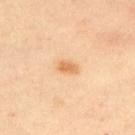biopsy status: imaged on a skin check; not biopsied | subject: male, aged around 45 | tile lighting: cross-polarized | location: the upper back | acquisition: 15 mm crop, total-body photography | TBP lesion metrics: a footprint of about 3 mm², a shape eccentricity near 0.85, and a symmetry-axis asymmetry near 0.3; a border-irregularity index near 2.5/10, a color-variation rating of about 1.5/10, and radial color variation of about 0.5; an automated nevus-likeness rating near 90 out of 100 and a detector confidence of about 100 out of 100 that the crop contains a lesion.